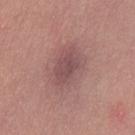This lesion was catalogued during total-body skin photography and was not selected for biopsy. The lesion's longest dimension is about 5.5 mm. Captured under white-light illumination. A female subject, roughly 25 years of age. Located on the right thigh. A 15 mm close-up tile from a total-body photography series done for melanoma screening.